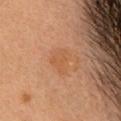{
  "biopsy_status": "not biopsied; imaged during a skin examination",
  "lighting": "cross-polarized",
  "image": {
    "source": "total-body photography crop",
    "field_of_view_mm": 15
  },
  "site": "head or neck",
  "automated_metrics": {
    "area_mm2_approx": 6.0,
    "eccentricity": 0.6,
    "shape_asymmetry": 0.35,
    "cielab_L": 50,
    "cielab_a": 22,
    "cielab_b": 35,
    "vs_skin_darker_L": 4.0,
    "vs_skin_contrast_norm": 4.5,
    "color_variation_0_10": 1.5,
    "peripheral_color_asymmetry": 0.5
  },
  "patient": {
    "sex": "female",
    "age_approx": 30
  }
}